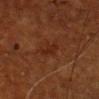| feature | finding |
|---|---|
| notes | no biopsy performed (imaged during a skin exam) |
| lesion diameter | ≈3.5 mm |
| site | the head or neck |
| lighting | cross-polarized illumination |
| acquisition | total-body-photography crop, ~15 mm field of view |
| patient | male, aged around 75 |
| automated lesion analysis | a lesion area of about 5.5 mm², an eccentricity of roughly 0.9, and a shape-asymmetry score of about 0.25 (0 = symmetric); a lesion color around L≈21 a*≈20 b*≈25 in CIELAB, a lesion–skin lightness drop of about 5, and a lesion-to-skin contrast of about 6 (normalized; higher = more distinct); a nevus-likeness score of about 0/100 and a detector confidence of about 100 out of 100 that the crop contains a lesion |